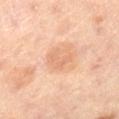biopsy status = imaged on a skin check; not biopsied | patient = female, aged 43–47 | site = the leg | acquisition = 15 mm crop, total-body photography.